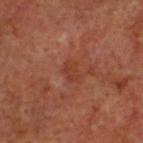Part of a total-body skin-imaging series; this lesion was reviewed on a skin check and was not flagged for biopsy. Imaged with cross-polarized lighting. This image is a 15 mm lesion crop taken from a total-body photograph. Approximately 2.5 mm at its widest. The lesion-visualizer software estimated an area of roughly 3.5 mm², a shape eccentricity near 0.75, and a symmetry-axis asymmetry near 0.35. The software also gave a lesion color around L≈38 a*≈26 b*≈30 in CIELAB and roughly 6 lightness units darker than nearby skin. The lesion is on the head or neck. A male patient, aged approximately 60.